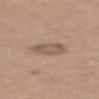| key | value |
|---|---|
| size | ~3 mm (longest diameter) |
| anatomic site | the upper back |
| image | total-body-photography crop, ~15 mm field of view |
| lighting | white-light illumination |
| patient | female, roughly 55 years of age |